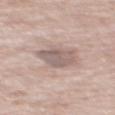Assessment:
This lesion was catalogued during total-body skin photography and was not selected for biopsy.
Acquisition and patient details:
Automated image analysis of the tile measured an average lesion color of about L≈59 a*≈15 b*≈19 (CIELAB), roughly 10 lightness units darker than nearby skin, and a lesion-to-skin contrast of about 7 (normalized; higher = more distinct). It also reported border irregularity of about 2.5 on a 0–10 scale, internal color variation of about 3 on a 0–10 scale, and radial color variation of about 1. And it measured a classifier nevus-likeness of about 0/100. Located on the upper back. Captured under white-light illumination. A female subject, about 75 years old. A roughly 15 mm field-of-view crop from a total-body skin photograph.